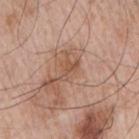Q: What is the anatomic site?
A: the right upper arm
Q: Lesion size?
A: ≈3 mm
Q: What is the imaging modality?
A: ~15 mm crop, total-body skin-cancer survey
Q: What are the patient's age and sex?
A: male, aged 63–67
Q: How was the tile lit?
A: white-light illumination